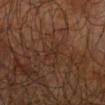follow-up = catalogued during a skin exam; not biopsied | lighting = cross-polarized illumination | patient = male, approximately 65 years of age | location = the right upper arm | acquisition = total-body-photography crop, ~15 mm field of view.